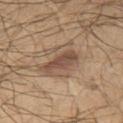The lesion was tiled from a total-body skin photograph and was not biopsied. The subject is a male roughly 50 years of age. The lesion is on the chest. A lesion tile, about 15 mm wide, cut from a 3D total-body photograph.